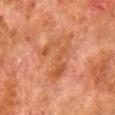This lesion was catalogued during total-body skin photography and was not selected for biopsy. The lesion is on the leg. The recorded lesion diameter is about 5 mm. A close-up tile cropped from a whole-body skin photograph, about 15 mm across. Captured under cross-polarized illumination. A male subject aged 78 to 82.